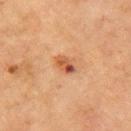No biopsy was performed on this lesion — it was imaged during a full skin examination and was not determined to be concerning.
The patient is a female aged 68 to 72.
Cropped from a total-body skin-imaging series; the visible field is about 15 mm.
On the right upper arm.